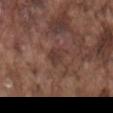workup: catalogued during a skin exam; not biopsied
patient: male, roughly 75 years of age
site: the mid back
lesion size: about 2.5 mm
lighting: white-light illumination
image source: total-body-photography crop, ~15 mm field of view